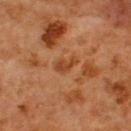Image and clinical context:
The patient is a male about 65 years old. An algorithmic analysis of the crop reported an area of roughly 3.5 mm², an eccentricity of roughly 0.85, and two-axis asymmetry of about 0.35. The software also gave border irregularity of about 3.5 on a 0–10 scale, a within-lesion color-variation index near 1/10, and radial color variation of about 0.5. The analysis additionally found a classifier nevus-likeness of about 0/100 and lesion-presence confidence of about 100/100. A roughly 15 mm field-of-view crop from a total-body skin photograph. Approximately 3 mm at its widest. Captured under cross-polarized illumination. From the upper back.